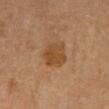- follow-up: no biopsy performed (imaged during a skin exam)
- image: 15 mm crop, total-body photography
- anatomic site: the mid back
- subject: male, approximately 60 years of age